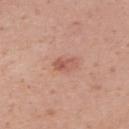Clinical impression: The lesion was tiled from a total-body skin photograph and was not biopsied. Image and clinical context: Captured under white-light illumination. The patient is a female approximately 40 years of age. On the upper back. The recorded lesion diameter is about 3 mm. A roughly 15 mm field-of-view crop from a total-body skin photograph.